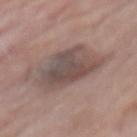Imaged during a routine full-body skin examination; the lesion was not biopsied and no histopathology is available.
The recorded lesion diameter is about 6 mm.
A close-up tile cropped from a whole-body skin photograph, about 15 mm across.
A female subject, in their mid-70s.
The lesion is located on the mid back.